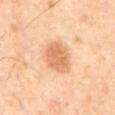{
  "biopsy_status": "not biopsied; imaged during a skin examination",
  "automated_metrics": {
    "area_mm2_approx": 11.0,
    "eccentricity": 0.6,
    "shape_asymmetry": 0.15,
    "border_irregularity_0_10": 1.5,
    "color_variation_0_10": 2.5,
    "peripheral_color_asymmetry": 1.0,
    "nevus_likeness_0_100": 90,
    "lesion_detection_confidence_0_100": 100
  },
  "lesion_size": {
    "long_diameter_mm_approx": 4.0
  },
  "image": {
    "source": "total-body photography crop",
    "field_of_view_mm": 15
  },
  "lighting": "cross-polarized",
  "site": "abdomen",
  "patient": {
    "sex": "male",
    "age_approx": 65
  }
}